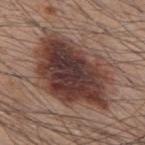Case summary:
- biopsy status — catalogued during a skin exam; not biopsied
- imaging modality — total-body-photography crop, ~15 mm field of view
- patient — male, about 60 years old
- site — the upper back